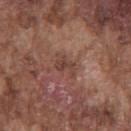Q: Is there a histopathology result?
A: total-body-photography surveillance lesion; no biopsy
Q: What is the anatomic site?
A: the chest
Q: What are the patient's age and sex?
A: male, in their mid- to late 70s
Q: What kind of image is this?
A: 15 mm crop, total-body photography
Q: Illumination type?
A: white-light
Q: What is the lesion's diameter?
A: ~2.5 mm (longest diameter)